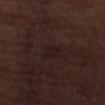workup: catalogued during a skin exam; not biopsied
location: the left lower leg
automated lesion analysis: a footprint of about 4.5 mm² and a shape-asymmetry score of about 0.3 (0 = symmetric)
patient: male, aged 68 to 72
image: ~15 mm crop, total-body skin-cancer survey
tile lighting: white-light illumination